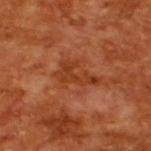{"biopsy_status": "not biopsied; imaged during a skin examination", "image": {"source": "total-body photography crop", "field_of_view_mm": 15}, "lesion_size": {"long_diameter_mm_approx": 4.5}, "patient": {"sex": "male", "age_approx": 65}, "lighting": "cross-polarized"}